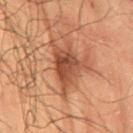{"biopsy_status": "not biopsied; imaged during a skin examination", "patient": {"sex": "male", "age_approx": 50}, "lesion_size": {"long_diameter_mm_approx": 5.0}, "site": "lower back", "lighting": "cross-polarized", "image": {"source": "total-body photography crop", "field_of_view_mm": 15}}